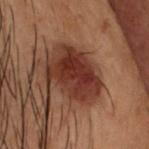biopsy status — catalogued during a skin exam; not biopsied
subject — male, in their mid- to late 50s
tile lighting — cross-polarized
diameter — ≈6 mm
site — the head or neck
acquisition — ~15 mm crop, total-body skin-cancer survey